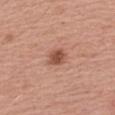Findings:
• workup · catalogued during a skin exam; not biopsied
• lesion size · ~2.5 mm (longest diameter)
• patient · female, aged approximately 65
• body site · the arm
• tile lighting · white-light illumination
• image source · total-body-photography crop, ~15 mm field of view
• image-analysis metrics · a lesion color around L≈52 a*≈25 b*≈30 in CIELAB, roughly 12 lightness units darker than nearby skin, and a lesion-to-skin contrast of about 8.5 (normalized; higher = more distinct); a border-irregularity index near 2/10, a color-variation rating of about 2/10, and peripheral color asymmetry of about 1